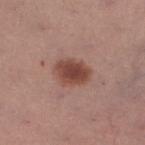The tile uses white-light illumination. Cropped from a total-body skin-imaging series; the visible field is about 15 mm. Longest diameter approximately 4 mm. A female patient, aged around 55. The lesion is located on the left lower leg. Automated tile analysis of the lesion measured a lesion area of about 9.5 mm², an outline eccentricity of about 0.7 (0 = round, 1 = elongated), and two-axis asymmetry of about 0.15. It also reported a mean CIELAB color near L≈45 a*≈23 b*≈25. The analysis additionally found a border-irregularity rating of about 1.5/10 and a within-lesion color-variation index near 3.5/10.